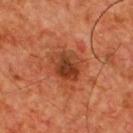biopsy_status: not biopsied; imaged during a skin examination
site: front of the torso
lighting: cross-polarized
automated_metrics:
  border_irregularity_0_10: 2.0
  color_variation_0_10: 5.5
  nevus_likeness_0_100: 5
  lesion_detection_confidence_0_100: 100
lesion_size:
  long_diameter_mm_approx: 4.0
image:
  source: total-body photography crop
  field_of_view_mm: 15
patient:
  sex: male
  age_approx: 55